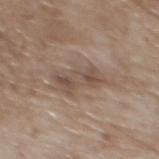Q: How was this image acquired?
A: ~15 mm crop, total-body skin-cancer survey
Q: What are the patient's age and sex?
A: male, in their 70s
Q: Where on the body is the lesion?
A: the mid back